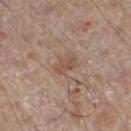No biopsy was performed on this lesion — it was imaged during a full skin examination and was not determined to be concerning. A male patient in their 70s. The total-body-photography lesion software estimated a footprint of about 3.5 mm². The software also gave an average lesion color of about L≈51 a*≈18 b*≈27 (CIELAB), a lesion–skin lightness drop of about 7, and a normalized lesion–skin contrast near 5.5. It also reported a border-irregularity rating of about 3.5/10, a within-lesion color-variation index near 1.5/10, and peripheral color asymmetry of about 0.5. The analysis additionally found a nevus-likeness score of about 0/100. Imaged with white-light lighting. This image is a 15 mm lesion crop taken from a total-body photograph. The lesion is located on the left lower leg.